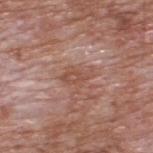Case summary:
• biopsy status — total-body-photography surveillance lesion; no biopsy
• lesion diameter — about 3.5 mm
• site — the upper back
• patient — male, aged 58–62
• imaging modality — ~15 mm tile from a whole-body skin photo
• automated lesion analysis — an area of roughly 5 mm² and a shape eccentricity near 0.8; a normalized border contrast of about 6; a border-irregularity rating of about 4.5/10 and peripheral color asymmetry of about 1; an automated nevus-likeness rating near 0 out of 100